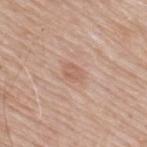  biopsy_status: not biopsied; imaged during a skin examination
  lighting: white-light
  image:
    source: total-body photography crop
    field_of_view_mm: 15
  site: upper back
  patient:
    sex: male
    age_approx: 65